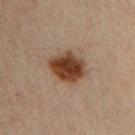The lesion was photographed on a routine skin check and not biopsied; there is no pathology result.
A male subject, aged 28–32.
The recorded lesion diameter is about 4.5 mm.
Located on the chest.
A 15 mm close-up extracted from a 3D total-body photography capture.
The lesion-visualizer software estimated a lesion area of about 12 mm² and an eccentricity of roughly 0.6. And it measured a mean CIELAB color near L≈33 a*≈17 b*≈26 and a normalized border contrast of about 13. The software also gave a nevus-likeness score of about 100/100 and a detector confidence of about 100 out of 100 that the crop contains a lesion.
The tile uses cross-polarized illumination.